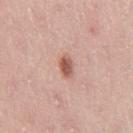The lesion was tiled from a total-body skin photograph and was not biopsied. Imaged with white-light lighting. The lesion is on the right thigh. A region of skin cropped from a whole-body photographic capture, roughly 15 mm wide. A female patient, about 40 years old.